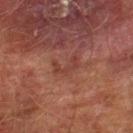The subject is a male about 75 years old. About 3 mm across. Cropped from a whole-body photographic skin survey; the tile spans about 15 mm. On the right lower leg.